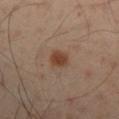{
  "biopsy_status": "not biopsied; imaged during a skin examination",
  "lesion_size": {
    "long_diameter_mm_approx": 2.5
  },
  "patient": {
    "sex": "male",
    "age_approx": 50
  },
  "site": "arm",
  "lighting": "cross-polarized",
  "image": {
    "source": "total-body photography crop",
    "field_of_view_mm": 15
  },
  "automated_metrics": {
    "area_mm2_approx": 5.5,
    "eccentricity": 0.35,
    "shape_asymmetry": 0.2,
    "cielab_L": 41,
    "cielab_a": 18,
    "cielab_b": 29,
    "vs_skin_darker_L": 9.0,
    "vs_skin_contrast_norm": 8.5,
    "nevus_likeness_0_100": 95,
    "lesion_detection_confidence_0_100": 100
  }
}